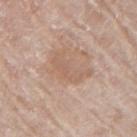Recorded during total-body skin imaging; not selected for excision or biopsy. A roughly 15 mm field-of-view crop from a total-body skin photograph. The patient is a male roughly 70 years of age. Automated image analysis of the tile measured an outline eccentricity of about 0.8 (0 = round, 1 = elongated). It also reported a mean CIELAB color near L≈60 a*≈17 b*≈28 and roughly 6 lightness units darker than nearby skin. And it measured an automated nevus-likeness rating near 0 out of 100 and lesion-presence confidence of about 100/100. The lesion is on the right upper arm. The recorded lesion diameter is about 4.5 mm. This is a white-light tile.